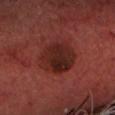Q: Is there a histopathology result?
A: no biopsy performed (imaged during a skin exam)
Q: Lesion location?
A: the head or neck
Q: What is the imaging modality?
A: 15 mm crop, total-body photography
Q: What are the patient's age and sex?
A: male, aged approximately 70
Q: What did automated image analysis measure?
A: an area of roughly 17 mm², a shape eccentricity near 0.55, and two-axis asymmetry of about 0.1; a mean CIELAB color near L≈24 a*≈24 b*≈23, roughly 9 lightness units darker than nearby skin, and a normalized lesion–skin contrast near 9; a nevus-likeness score of about 95/100 and a lesion-detection confidence of about 100/100
Q: How large is the lesion?
A: ≈5 mm
Q: Illumination type?
A: cross-polarized illumination